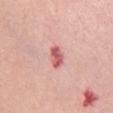{
  "site": "front of the torso",
  "image": {
    "source": "total-body photography crop",
    "field_of_view_mm": 15
  },
  "lesion_size": {
    "long_diameter_mm_approx": 3.0
  },
  "patient": {
    "sex": "female",
    "age_approx": 55
  },
  "lighting": "white-light"
}